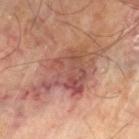biopsy status=imaged on a skin check; not biopsied | automated metrics=a border-irregularity rating of about 6/10 and a color-variation rating of about 8/10; an automated nevus-likeness rating near 0 out of 100 and a detector confidence of about 100 out of 100 that the crop contains a lesion | subject=male, approximately 70 years of age | body site=the left thigh | image=~15 mm tile from a whole-body skin photo | lighting=cross-polarized.